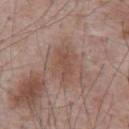Clinical summary:
The lesion-visualizer software estimated border irregularity of about 2.5 on a 0–10 scale, a within-lesion color-variation index near 3/10, and peripheral color asymmetry of about 1. The patient is a male roughly 70 years of age. Cropped from a total-body skin-imaging series; the visible field is about 15 mm. Imaged with white-light lighting. About 5 mm across. The lesion is on the abdomen.